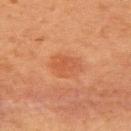Part of a total-body skin-imaging series; this lesion was reviewed on a skin check and was not flagged for biopsy. A female patient aged 48 to 52. The tile uses cross-polarized illumination. From the upper back. The lesion's longest dimension is about 3.5 mm. A roughly 15 mm field-of-view crop from a total-body skin photograph.